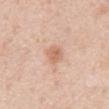Notes:
• follow-up · imaged on a skin check; not biopsied
• lighting · white-light illumination
• location · the abdomen
• subject · male, aged 53 to 57
• lesion diameter · ~2.5 mm (longest diameter)
• image · total-body-photography crop, ~15 mm field of view